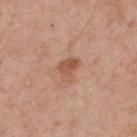biopsy status — catalogued during a skin exam; not biopsied | imaging modality — ~15 mm tile from a whole-body skin photo | diameter — ~3 mm (longest diameter) | subject — male, in their mid- to late 50s | body site — the upper back | lighting — white-light illumination | TBP lesion metrics — an area of roughly 4.5 mm², an eccentricity of roughly 0.7, and a symmetry-axis asymmetry near 0.4; a normalized lesion–skin contrast near 7; a border-irregularity index near 3.5/10 and internal color variation of about 4 on a 0–10 scale.